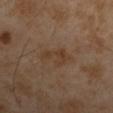| field | value |
|---|---|
| follow-up | imaged on a skin check; not biopsied |
| lesion diameter | ≈3.5 mm |
| image | ~15 mm tile from a whole-body skin photo |
| site | the left upper arm |
| subject | male, aged approximately 55 |